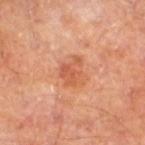Q: Was this lesion biopsied?
A: total-body-photography surveillance lesion; no biopsy
Q: What is the anatomic site?
A: the leg
Q: What are the patient's age and sex?
A: male, roughly 70 years of age
Q: What lighting was used for the tile?
A: cross-polarized illumination
Q: What is the imaging modality?
A: ~15 mm crop, total-body skin-cancer survey